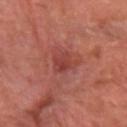Part of a total-body skin-imaging series; this lesion was reviewed on a skin check and was not flagged for biopsy. A region of skin cropped from a whole-body photographic capture, roughly 15 mm wide. This is a cross-polarized tile. The lesion-visualizer software estimated an outline eccentricity of about 0.7 (0 = round, 1 = elongated). It also reported a mean CIELAB color near L≈41 a*≈30 b*≈26 and roughly 8 lightness units darker than nearby skin. The software also gave a classifier nevus-likeness of about 20/100 and a detector confidence of about 100 out of 100 that the crop contains a lesion. A patient aged 63 to 67. The lesion is on the left forearm.